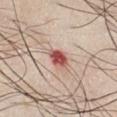<lesion>
<biopsy_status>not biopsied; imaged during a skin examination</biopsy_status>
<automated_metrics>
  <area_mm2_approx>4.5</area_mm2_approx>
  <eccentricity>0.6</eccentricity>
  <cielab_L>54</cielab_L>
  <cielab_a>29</cielab_a>
  <cielab_b>26</cielab_b>
  <vs_skin_darker_L>17.0</vs_skin_darker_L>
  <vs_skin_contrast_norm>11.0</vs_skin_contrast_norm>
  <border_irregularity_0_10>1.5</border_irregularity_0_10>
  <color_variation_0_10>6.5</color_variation_0_10>
  <peripheral_color_asymmetry>2.5</peripheral_color_asymmetry>
</automated_metrics>
<patient>
  <sex>male</sex>
  <age_approx>45</age_approx>
</patient>
<site>front of the torso</site>
<lighting>white-light</lighting>
<image>
  <source>total-body photography crop</source>
  <field_of_view_mm>15</field_of_view_mm>
</image>
<lesion_size>
  <long_diameter_mm_approx>2.5</long_diameter_mm_approx>
</lesion_size>
</lesion>